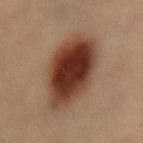The lesion was tiled from a total-body skin photograph and was not biopsied.
This is a cross-polarized tile.
On the lower back.
The total-body-photography lesion software estimated a lesion area of about 33 mm², an outline eccentricity of about 0.5 (0 = round, 1 = elongated), and a shape-asymmetry score of about 0.15 (0 = symmetric). The analysis additionally found an average lesion color of about L≈32 a*≈19 b*≈26 (CIELAB) and about 16 CIELAB-L* units darker than the surrounding skin. The software also gave a border-irregularity rating of about 1.5/10, internal color variation of about 7 on a 0–10 scale, and radial color variation of about 1.5. It also reported a nevus-likeness score of about 100/100 and lesion-presence confidence of about 100/100.
About 7 mm across.
A female subject, aged approximately 60.
Cropped from a whole-body photographic skin survey; the tile spans about 15 mm.